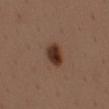Imaged during a routine full-body skin examination; the lesion was not biopsied and no histopathology is available. An algorithmic analysis of the crop reported a nevus-likeness score of about 100/100 and a lesion-detection confidence of about 100/100. Approximately 3.5 mm at its widest. Captured under white-light illumination. A male patient, about 40 years old. On the mid back. Cropped from a whole-body photographic skin survey; the tile spans about 15 mm.